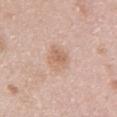Case summary:
• biopsy status — catalogued during a skin exam; not biopsied
• subject — female, aged 48–52
• illumination — white-light
• site — the left upper arm
• image source — ~15 mm tile from a whole-body skin photo